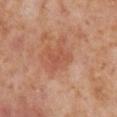Notes:
* follow-up — catalogued during a skin exam; not biopsied
* image-analysis metrics — a mean CIELAB color near L≈53 a*≈26 b*≈33, roughly 6 lightness units darker than nearby skin, and a normalized border contrast of about 4.5
* image — 15 mm crop, total-body photography
* diameter — about 3 mm
* patient — female, aged 53–57
* lighting — cross-polarized illumination
* site — the leg